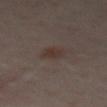– follow-up — catalogued during a skin exam; not biopsied
– diameter — about 2.5 mm
– body site — the front of the torso
– imaging modality — ~15 mm tile from a whole-body skin photo
– lighting — cross-polarized
– patient — aged 53 to 57